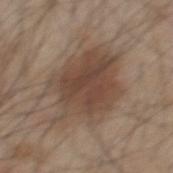{
  "patient": {
    "sex": "male",
    "age_approx": 45
  },
  "site": "mid back",
  "image": {
    "source": "total-body photography crop",
    "field_of_view_mm": 15
  }
}